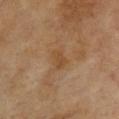Assessment:
The lesion was photographed on a routine skin check and not biopsied; there is no pathology result.
Clinical summary:
This image is a 15 mm lesion crop taken from a total-body photograph. The patient is a female approximately 60 years of age. The tile uses cross-polarized illumination. The lesion is located on the chest. About 2.5 mm across.